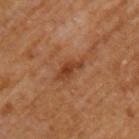{"image": {"source": "total-body photography crop", "field_of_view_mm": 15}, "patient": {"sex": "male", "age_approx": 65}, "automated_metrics": {"border_irregularity_0_10": 4.0, "peripheral_color_asymmetry": 0.5, "nevus_likeness_0_100": 50, "lesion_detection_confidence_0_100": 100}, "lesion_size": {"long_diameter_mm_approx": 3.5}, "site": "left upper arm"}